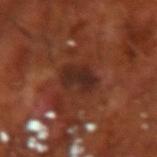Clinical impression:
Imaged during a routine full-body skin examination; the lesion was not biopsied and no histopathology is available.
Context:
On the arm. A male subject, roughly 70 years of age. The tile uses cross-polarized illumination. A 15 mm close-up tile from a total-body photography series done for melanoma screening. Longest diameter approximately 4 mm.